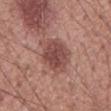Part of a total-body skin-imaging series; this lesion was reviewed on a skin check and was not flagged for biopsy.
From the mid back.
This is a white-light tile.
A male subject, in their mid-50s.
A 15 mm close-up extracted from a 3D total-body photography capture.
The recorded lesion diameter is about 5.5 mm.
An algorithmic analysis of the crop reported a lesion area of about 15 mm² and an outline eccentricity of about 0.75 (0 = round, 1 = elongated). The software also gave lesion-presence confidence of about 100/100.